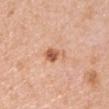Case summary:
– notes: no biopsy performed (imaged during a skin exam)